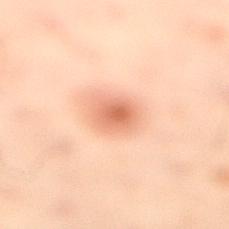Part of a total-body skin-imaging series; this lesion was reviewed on a skin check and was not flagged for biopsy. Captured under cross-polarized illumination. A 15 mm close-up extracted from a 3D total-body photography capture. A female subject roughly 30 years of age. Automated tile analysis of the lesion measured an area of roughly 7.5 mm² and a shape eccentricity near 0.55. It also reported a lesion color around L≈55 a*≈22 b*≈29 in CIELAB, about 10 CIELAB-L* units darker than the surrounding skin, and a lesion-to-skin contrast of about 7 (normalized; higher = more distinct). The analysis additionally found a border-irregularity index near 1/10 and a color-variation rating of about 4/10. The lesion is located on the left leg. About 3 mm across.